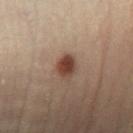Captured during whole-body skin photography for melanoma surveillance; the lesion was not biopsied. Measured at roughly 3 mm in maximum diameter. From the left leg. Automated tile analysis of the lesion measured a footprint of about 5.5 mm² and an outline eccentricity of about 0.7 (0 = round, 1 = elongated). The software also gave a lesion color around L≈33 a*≈16 b*≈21 in CIELAB and roughly 11 lightness units darker than nearby skin. The subject is a male aged around 50. Captured under cross-polarized illumination. This image is a 15 mm lesion crop taken from a total-body photograph.